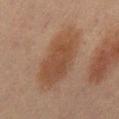- follow-up: imaged on a skin check; not biopsied
- anatomic site: the front of the torso
- subject: male, aged around 65
- image: 15 mm crop, total-body photography